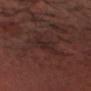biopsy status=no biopsy performed (imaged during a skin exam) | imaging modality=~15 mm crop, total-body skin-cancer survey | patient=male, approximately 35 years of age | image-analysis metrics=a border-irregularity rating of about 4.5/10 and a within-lesion color-variation index near 1.5/10; a lesion-detection confidence of about 50/100 | lighting=cross-polarized | body site=the head or neck.